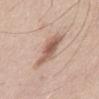The lesion was tiled from a total-body skin photograph and was not biopsied.
A roughly 15 mm field-of-view crop from a total-body skin photograph.
The lesion is located on the abdomen.
The subject is a male aged 48–52.
About 5 mm across.